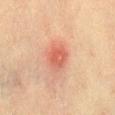lesion size: ≈3 mm | automated metrics: an average lesion color of about L≈52 a*≈28 b*≈29 (CIELAB), about 9 CIELAB-L* units darker than the surrounding skin, and a normalized lesion–skin contrast near 6.5; border irregularity of about 2 on a 0–10 scale and internal color variation of about 4 on a 0–10 scale; a classifier nevus-likeness of about 0/100 and a detector confidence of about 100 out of 100 that the crop contains a lesion | location: the abdomen | tile lighting: cross-polarized | subject: female, aged around 40 | imaging modality: ~15 mm tile from a whole-body skin photo.